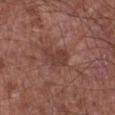<lesion>
<biopsy_status>not biopsied; imaged during a skin examination</biopsy_status>
<automated_metrics>
  <area_mm2_approx>5.0</area_mm2_approx>
  <eccentricity>0.8</eccentricity>
  <shape_asymmetry>0.4</shape_asymmetry>
  <border_irregularity_0_10>4.5</border_irregularity_0_10>
  <color_variation_0_10>2.0</color_variation_0_10>
  <nevus_likeness_0_100>0</nevus_likeness_0_100>
  <lesion_detection_confidence_0_100>100</lesion_detection_confidence_0_100>
</automated_metrics>
<patient>
  <sex>male</sex>
  <age_approx>65</age_approx>
</patient>
<image>
  <source>total-body photography crop</source>
  <field_of_view_mm>15</field_of_view_mm>
</image>
<site>right lower leg</site>
</lesion>